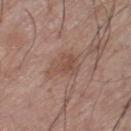No biopsy was performed on this lesion — it was imaged during a full skin examination and was not determined to be concerning. The lesion is located on the leg. The subject is a male about 70 years old. Measured at roughly 3.5 mm in maximum diameter. A roughly 15 mm field-of-view crop from a total-body skin photograph. An algorithmic analysis of the crop reported an area of roughly 6.5 mm², an outline eccentricity of about 0.7 (0 = round, 1 = elongated), and two-axis asymmetry of about 0.5. The software also gave a lesion color around L≈49 a*≈19 b*≈26 in CIELAB, a lesion–skin lightness drop of about 8, and a normalized border contrast of about 6. The software also gave a color-variation rating of about 2.5/10. This is a white-light tile.